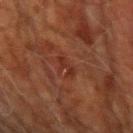workup = catalogued during a skin exam; not biopsied | anatomic site = the right upper arm | imaging modality = ~15 mm crop, total-body skin-cancer survey | TBP lesion metrics = a border-irregularity index near 5.5/10, a within-lesion color-variation index near 0/10, and radial color variation of about 0 | patient = male, in their 70s | diameter = ~3 mm (longest diameter).